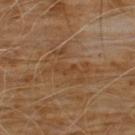Assessment: This lesion was catalogued during total-body skin photography and was not selected for biopsy. Acquisition and patient details: A male subject, aged 58 to 62. Automated tile analysis of the lesion measured a footprint of about 13 mm², an eccentricity of roughly 0.8, and a shape-asymmetry score of about 0.35 (0 = symmetric). And it measured a lesion color around L≈41 a*≈17 b*≈32 in CIELAB and roughly 5 lightness units darker than nearby skin. The software also gave a within-lesion color-variation index near 3/10. A 15 mm crop from a total-body photograph taken for skin-cancer surveillance. From the chest.